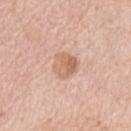notes: total-body-photography surveillance lesion; no biopsy